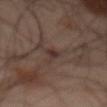Imaged during a routine full-body skin examination; the lesion was not biopsied and no histopathology is available. A region of skin cropped from a whole-body photographic capture, roughly 15 mm wide. Located on the abdomen. Captured under cross-polarized illumination. A male patient, roughly 65 years of age. Longest diameter approximately 2.5 mm.